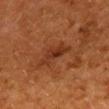biopsy status=catalogued during a skin exam; not biopsied | tile lighting=cross-polarized illumination | subject=female, aged 48–52 | automated lesion analysis=a footprint of about 7 mm²; a classifier nevus-likeness of about 20/100 | acquisition=~15 mm tile from a whole-body skin photo | site=the mid back.